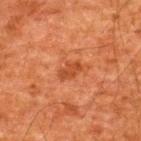follow-up — catalogued during a skin exam; not biopsied
automated metrics — an outline eccentricity of about 0.8 (0 = round, 1 = elongated) and a shape-asymmetry score of about 0.3 (0 = symmetric); an average lesion color of about L≈38 a*≈26 b*≈34 (CIELAB), about 7 CIELAB-L* units darker than the surrounding skin, and a lesion-to-skin contrast of about 6.5 (normalized; higher = more distinct); border irregularity of about 3 on a 0–10 scale, internal color variation of about 1 on a 0–10 scale, and peripheral color asymmetry of about 0.5; a detector confidence of about 100 out of 100 that the crop contains a lesion
patient — male, about 60 years old
anatomic site — the upper back
illumination — cross-polarized illumination
lesion diameter — ≈3 mm
acquisition — ~15 mm tile from a whole-body skin photo